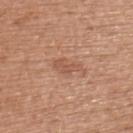follow-up = no biopsy performed (imaged during a skin exam) | patient = female, approximately 65 years of age | image = total-body-photography crop, ~15 mm field of view | TBP lesion metrics = an area of roughly 4.5 mm² and a shape eccentricity near 0.85; a border-irregularity index near 4/10 and a within-lesion color-variation index near 1.5/10; an automated nevus-likeness rating near 10 out of 100 and a detector confidence of about 100 out of 100 that the crop contains a lesion | site = the upper back | diameter = ~3.5 mm (longest diameter) | illumination = white-light.